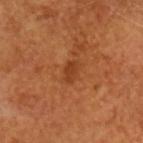automated_metrics:
  lesion_detection_confidence_0_100: 100
lesion_size:
  long_diameter_mm_approx: 2.5
image:
  source: total-body photography crop
  field_of_view_mm: 15
patient:
  sex: male
  age_approx: 65
site: head or neck